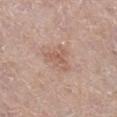patient=female, in their mid-60s
acquisition=total-body-photography crop, ~15 mm field of view
lesion diameter=≈3.5 mm
automated metrics=a lesion color around L≈58 a*≈20 b*≈27 in CIELAB, a lesion–skin lightness drop of about 7, and a normalized border contrast of about 5; border irregularity of about 5.5 on a 0–10 scale, a within-lesion color-variation index near 3/10, and a peripheral color-asymmetry measure near 1; a detector confidence of about 100 out of 100 that the crop contains a lesion
anatomic site=the leg